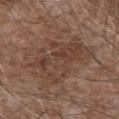biopsy status = total-body-photography surveillance lesion; no biopsy
patient = male, aged 58 to 62
site = the mid back
illumination = white-light
image = 15 mm crop, total-body photography